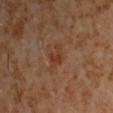Q: Was this lesion biopsied?
A: catalogued during a skin exam; not biopsied
Q: What is the imaging modality?
A: ~15 mm tile from a whole-body skin photo
Q: Who is the patient?
A: male, aged 58 to 62
Q: How was the tile lit?
A: cross-polarized illumination
Q: What is the anatomic site?
A: the chest